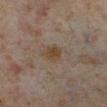This lesion was catalogued during total-body skin photography and was not selected for biopsy. On the right lower leg. A lesion tile, about 15 mm wide, cut from a 3D total-body photograph. Captured under cross-polarized illumination. Automated tile analysis of the lesion measured a footprint of about 4.5 mm², a shape eccentricity near 0.65, and a symmetry-axis asymmetry near 0.25. The software also gave a classifier nevus-likeness of about 15/100 and lesion-presence confidence of about 100/100. The patient is a female approximately 50 years of age.